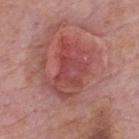The lesion was tiled from a total-body skin photograph and was not biopsied. The tile uses white-light illumination. On the upper back. A male subject aged around 75. An algorithmic analysis of the crop reported a shape-asymmetry score of about 0.3 (0 = symmetric). The analysis additionally found a mean CIELAB color near L≈48 a*≈30 b*≈24, about 9 CIELAB-L* units darker than the surrounding skin, and a lesion-to-skin contrast of about 6.5 (normalized; higher = more distinct). And it measured border irregularity of about 4.5 on a 0–10 scale and a peripheral color-asymmetry measure near 2.5. The software also gave a classifier nevus-likeness of about 0/100 and a lesion-detection confidence of about 100/100. A lesion tile, about 15 mm wide, cut from a 3D total-body photograph. Longest diameter approximately 6.5 mm.